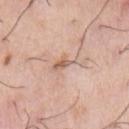notes: no biopsy performed (imaged during a skin exam); location: the chest; image-analysis metrics: a classifier nevus-likeness of about 0/100 and lesion-presence confidence of about 80/100; image: 15 mm crop, total-body photography; subject: male, in their mid-70s.